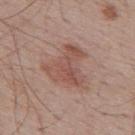{"automated_metrics": {"cielab_L": 52, "cielab_a": 21, "cielab_b": 25, "vs_skin_darker_L": 8.0, "vs_skin_contrast_norm": 6.0, "border_irregularity_0_10": 6.0, "peripheral_color_asymmetry": 1.5, "nevus_likeness_0_100": 35}, "image": {"source": "total-body photography crop", "field_of_view_mm": 15}, "site": "upper back", "patient": {"sex": "male", "age_approx": 70}, "lighting": "white-light"}